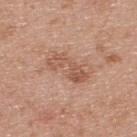<lesion>
<biopsy_status>not biopsied; imaged during a skin examination</biopsy_status>
<patient>
  <sex>male</sex>
  <age_approx>30</age_approx>
</patient>
<site>upper back</site>
<lighting>white-light</lighting>
<lesion_size>
  <long_diameter_mm_approx>5.0</long_diameter_mm_approx>
</lesion_size>
<image>
  <source>total-body photography crop</source>
  <field_of_view_mm>15</field_of_view_mm>
</image>
</lesion>